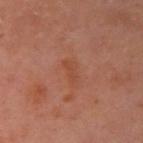acquisition: ~15 mm crop, total-body skin-cancer survey | patient: female, aged approximately 50 | body site: the left upper arm | image-analysis metrics: a mean CIELAB color near L≈45 a*≈25 b*≈32, roughly 5 lightness units darker than nearby skin, and a normalized border contrast of about 5; border irregularity of about 2.5 on a 0–10 scale, a color-variation rating of about 1.5/10, and peripheral color asymmetry of about 0.5.